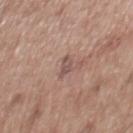The lesion was photographed on a routine skin check and not biopsied; there is no pathology result. Located on the mid back. A 15 mm close-up extracted from a 3D total-body photography capture. This is a white-light tile. A male subject in their 50s. The recorded lesion diameter is about 2.5 mm.